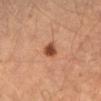Q: Was a biopsy performed?
A: no biopsy performed (imaged during a skin exam)
Q: Lesion size?
A: ≈2.5 mm
Q: What did automated image analysis measure?
A: a shape eccentricity near 0.55 and a symmetry-axis asymmetry near 0.25; an average lesion color of about L≈41 a*≈23 b*≈31 (CIELAB), about 14 CIELAB-L* units darker than the surrounding skin, and a lesion-to-skin contrast of about 10.5 (normalized; higher = more distinct); a border-irregularity index near 2.5/10, a within-lesion color-variation index near 2/10, and peripheral color asymmetry of about 0.5; an automated nevus-likeness rating near 100 out of 100 and lesion-presence confidence of about 100/100
Q: What lighting was used for the tile?
A: cross-polarized illumination
Q: What kind of image is this?
A: 15 mm crop, total-body photography
Q: Patient demographics?
A: male, aged 63 to 67
Q: Where on the body is the lesion?
A: the abdomen